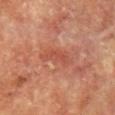Q: Was this lesion biopsied?
A: catalogued during a skin exam; not biopsied
Q: What is the anatomic site?
A: the chest
Q: What is the imaging modality?
A: 15 mm crop, total-body photography
Q: Automated lesion metrics?
A: an area of roughly 7 mm² and an outline eccentricity of about 0.9 (0 = round, 1 = elongated); a lesion color around L≈47 a*≈27 b*≈30 in CIELAB and a normalized lesion–skin contrast near 5
Q: Illumination type?
A: cross-polarized
Q: Lesion size?
A: ≈4.5 mm
Q: What are the patient's age and sex?
A: female, in their 80s